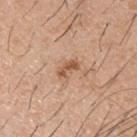Q: Was a biopsy performed?
A: total-body-photography surveillance lesion; no biopsy
Q: Lesion size?
A: ≈2.5 mm
Q: What are the patient's age and sex?
A: male, aged 38 to 42
Q: Lesion location?
A: the back
Q: How was the tile lit?
A: white-light illumination
Q: What is the imaging modality?
A: total-body-photography crop, ~15 mm field of view